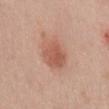biopsy status: total-body-photography surveillance lesion; no biopsy | subject: male, aged 33–37 | lighting: white-light illumination | body site: the mid back | image source: 15 mm crop, total-body photography.